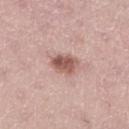This lesion was catalogued during total-body skin photography and was not selected for biopsy.